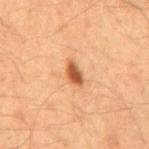Case summary:
– follow-up: total-body-photography surveillance lesion; no biopsy
– body site: the mid back
– tile lighting: cross-polarized illumination
– imaging modality: ~15 mm tile from a whole-body skin photo
– patient: male, aged 63–67
– size: about 3 mm
– automated metrics: an area of roughly 4 mm², a shape eccentricity near 0.85, and a symmetry-axis asymmetry near 0.25; a within-lesion color-variation index near 3/10; a lesion-detection confidence of about 100/100